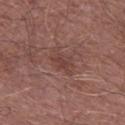| feature | finding |
|---|---|
| biopsy status | no biopsy performed (imaged during a skin exam) |
| imaging modality | ~15 mm crop, total-body skin-cancer survey |
| subject | male, aged 68–72 |
| location | the right lower leg |
| TBP lesion metrics | a footprint of about 5 mm², an eccentricity of roughly 0.8, and a shape-asymmetry score of about 0.4 (0 = symmetric); border irregularity of about 4.5 on a 0–10 scale, a within-lesion color-variation index near 1.5/10, and radial color variation of about 0.5; an automated nevus-likeness rating near 0 out of 100 |